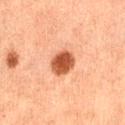Automated tile analysis of the lesion measured about 15 CIELAB-L* units darker than the surrounding skin and a normalized lesion–skin contrast near 11. And it measured a border-irregularity index near 1.5/10. The software also gave an automated nevus-likeness rating near 100 out of 100 and a lesion-detection confidence of about 100/100.
The subject is a female about 60 years old.
About 3 mm across.
A 15 mm crop from a total-body photograph taken for skin-cancer surveillance.
The tile uses cross-polarized illumination.
From the right thigh.